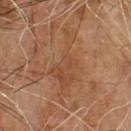No biopsy was performed on this lesion — it was imaged during a full skin examination and was not determined to be concerning. A 15 mm close-up tile from a total-body photography series done for melanoma screening. The tile uses cross-polarized illumination. A male patient aged 63–67. The recorded lesion diameter is about 8.5 mm. Automated tile analysis of the lesion measured a nevus-likeness score of about 0/100 and a lesion-detection confidence of about 70/100.